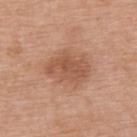This lesion was catalogued during total-body skin photography and was not selected for biopsy.
From the upper back.
The recorded lesion diameter is about 5 mm.
The tile uses white-light illumination.
A female patient, roughly 65 years of age.
A 15 mm close-up extracted from a 3D total-body photography capture.